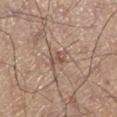Q: Was a biopsy performed?
A: total-body-photography surveillance lesion; no biopsy
Q: What is the anatomic site?
A: the right lower leg
Q: What are the patient's age and sex?
A: male, aged approximately 55
Q: Lesion size?
A: ~2.5 mm (longest diameter)
Q: What did automated image analysis measure?
A: a symmetry-axis asymmetry near 0.35; a border-irregularity index near 3.5/10, internal color variation of about 1.5 on a 0–10 scale, and a peripheral color-asymmetry measure near 0.5; lesion-presence confidence of about 60/100
Q: Illumination type?
A: white-light
Q: What kind of image is this?
A: ~15 mm crop, total-body skin-cancer survey